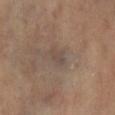A 15 mm close-up tile from a total-body photography series done for melanoma screening. Imaged with cross-polarized lighting. A female patient about 70 years old. From the right lower leg.